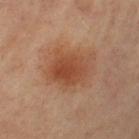Clinical impression:
No biopsy was performed on this lesion — it was imaged during a full skin examination and was not determined to be concerning.
Context:
Cropped from a whole-body photographic skin survey; the tile spans about 15 mm. Longest diameter approximately 5 mm. The patient is a female aged approximately 65. From the left upper arm.